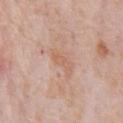biopsy_status: not biopsied; imaged during a skin examination
site: chest
image:
  source: total-body photography crop
  field_of_view_mm: 15
lighting: white-light
lesion_size:
  long_diameter_mm_approx: 3.0
patient:
  sex: male
  age_approx: 80